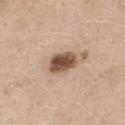Assessment:
The lesion was photographed on a routine skin check and not biopsied; there is no pathology result.
Clinical summary:
An algorithmic analysis of the crop reported a color-variation rating of about 5.5/10. It also reported a nevus-likeness score of about 100/100 and a detector confidence of about 100 out of 100 that the crop contains a lesion. Captured under white-light illumination. A female subject, in their mid- to late 40s. The lesion is located on the chest. The recorded lesion diameter is about 3.5 mm. A 15 mm close-up extracted from a 3D total-body photography capture.